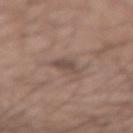A region of skin cropped from a whole-body photographic capture, roughly 15 mm wide. A male patient in their mid-50s.